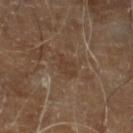Captured during whole-body skin photography for melanoma surveillance; the lesion was not biopsied.
Cropped from a whole-body photographic skin survey; the tile spans about 15 mm.
On the left lower leg.
The tile uses cross-polarized illumination.
A male patient aged 68 to 72.